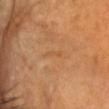Captured during whole-body skin photography for melanoma surveillance; the lesion was not biopsied.
A female patient approximately 70 years of age.
Automated image analysis of the tile measured an area of roughly 2 mm² and an outline eccentricity of about 0.9 (0 = round, 1 = elongated). The analysis additionally found an average lesion color of about L≈52 a*≈21 b*≈38 (CIELAB), about 4 CIELAB-L* units darker than the surrounding skin, and a lesion-to-skin contrast of about 3 (normalized; higher = more distinct). The software also gave a border-irregularity rating of about 3/10, internal color variation of about 0 on a 0–10 scale, and peripheral color asymmetry of about 0.
Located on the head or neck.
A region of skin cropped from a whole-body photographic capture, roughly 15 mm wide.
This is a cross-polarized tile.